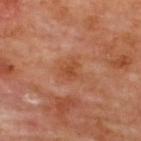No biopsy was performed on this lesion — it was imaged during a full skin examination and was not determined to be concerning. A 15 mm close-up tile from a total-body photography series done for melanoma screening. Captured under cross-polarized illumination. The patient is a male in their mid- to late 60s. About 2.5 mm across. The lesion is on the upper back.